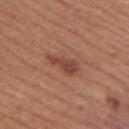Case summary:
• notes — imaged on a skin check; not biopsied
• diameter — ~4.5 mm (longest diameter)
• patient — male, aged approximately 55
• location — the upper back
• image — ~15 mm tile from a whole-body skin photo
• illumination — white-light